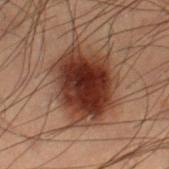Notes:
• follow-up — catalogued during a skin exam; not biopsied
• patient — male, aged 53–57
• tile lighting — cross-polarized
• acquisition — ~15 mm tile from a whole-body skin photo
• body site — the right lower leg